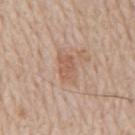This lesion was catalogued during total-body skin photography and was not selected for biopsy. Captured under white-light illumination. A lesion tile, about 15 mm wide, cut from a 3D total-body photograph. From the mid back. Automated image analysis of the tile measured an average lesion color of about L≈58 a*≈20 b*≈29 (CIELAB), roughly 8 lightness units darker than nearby skin, and a normalized border contrast of about 5.5. The software also gave a detector confidence of about 100 out of 100 that the crop contains a lesion. A male subject, in their 80s. About 4 mm across.